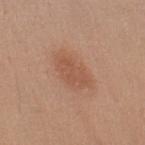| feature | finding |
|---|---|
| follow-up | total-body-photography surveillance lesion; no biopsy |
| imaging modality | 15 mm crop, total-body photography |
| site | the right upper arm |
| lighting | white-light illumination |
| subject | male, about 55 years old |